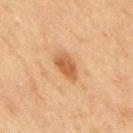Q: Was this lesion biopsied?
A: no biopsy performed (imaged during a skin exam)